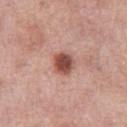– diameter · ≈3 mm
– acquisition · 15 mm crop, total-body photography
– body site · the left thigh
– patient · female, approximately 50 years of age
– image-analysis metrics · an area of roughly 6.5 mm² and an outline eccentricity of about 0.55 (0 = round, 1 = elongated); a mean CIELAB color near L≈50 a*≈25 b*≈27, roughly 16 lightness units darker than nearby skin, and a normalized border contrast of about 11; a border-irregularity index near 1.5/10, a color-variation rating of about 3.5/10, and a peripheral color-asymmetry measure near 1; a lesion-detection confidence of about 100/100
– tile lighting · white-light illumination